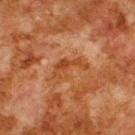{
  "biopsy_status": "not biopsied; imaged during a skin examination",
  "image": {
    "source": "total-body photography crop",
    "field_of_view_mm": 15
  },
  "lesion_size": {
    "long_diameter_mm_approx": 4.5
  },
  "lighting": "cross-polarized",
  "patient": {
    "sex": "male",
    "age_approx": 80
  },
  "automated_metrics": {
    "eccentricity": 0.9,
    "shape_asymmetry": 0.5
  },
  "site": "upper back"
}